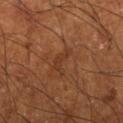patient:
  sex: male
  age_approx: 65
automated_metrics:
  border_irregularity_0_10: 8.0
  color_variation_0_10: 0.0
  peripheral_color_asymmetry: 0.0
  nevus_likeness_0_100: 0
  lesion_detection_confidence_0_100: 100
lesion_size:
  long_diameter_mm_approx: 3.5
image:
  source: total-body photography crop
  field_of_view_mm: 15
site: right lower leg